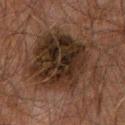The lesion was tiled from a total-body skin photograph and was not biopsied. The lesion-visualizer software estimated a mean CIELAB color near L≈22 a*≈12 b*≈19, roughly 9 lightness units darker than nearby skin, and a normalized border contrast of about 10.5. And it measured a border-irregularity index near 5.5/10, a within-lesion color-variation index near 6/10, and peripheral color asymmetry of about 2. Captured under cross-polarized illumination. A male patient, approximately 60 years of age. From the chest. A lesion tile, about 15 mm wide, cut from a 3D total-body photograph.